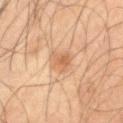Clinical impression: Captured during whole-body skin photography for melanoma surveillance; the lesion was not biopsied. Background: A region of skin cropped from a whole-body photographic capture, roughly 15 mm wide. About 4 mm across. The lesion is located on the right upper arm. The subject is a male roughly 65 years of age. The lesion-visualizer software estimated a footprint of about 7 mm², an outline eccentricity of about 0.8 (0 = round, 1 = elongated), and a symmetry-axis asymmetry near 0.25. The software also gave a lesion-detection confidence of about 100/100.